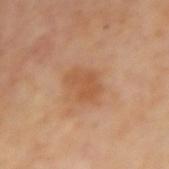workup = no biopsy performed (imaged during a skin exam)
patient = female, aged 53–57
site = the right forearm
acquisition = total-body-photography crop, ~15 mm field of view
automated lesion analysis = a lesion color around L≈55 a*≈23 b*≈37 in CIELAB and about 7 CIELAB-L* units darker than the surrounding skin; a color-variation rating of about 2.5/10 and a peripheral color-asymmetry measure near 1